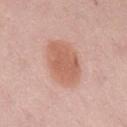Q: Is there a histopathology result?
A: no biopsy performed (imaged during a skin exam)